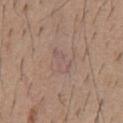No biopsy was performed on this lesion — it was imaged during a full skin examination and was not determined to be concerning. A male patient approximately 60 years of age. The lesion is on the chest. Cropped from a whole-body photographic skin survey; the tile spans about 15 mm. This is a white-light tile.